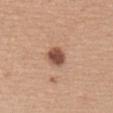Impression:
The lesion was tiled from a total-body skin photograph and was not biopsied.
Acquisition and patient details:
The lesion is on the arm. Cropped from a total-body skin-imaging series; the visible field is about 15 mm. The subject is a female in their mid- to late 30s.